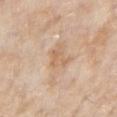follow-up = imaged on a skin check; not biopsied
location = the right upper arm
imaging modality = ~15 mm tile from a whole-body skin photo
subject = male, approximately 75 years of age